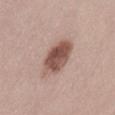{
  "lighting": "white-light",
  "image": {
    "source": "total-body photography crop",
    "field_of_view_mm": 15
  },
  "lesion_size": {
    "long_diameter_mm_approx": 4.5
  },
  "site": "left thigh",
  "patient": {
    "sex": "male",
    "age_approx": 45
  }
}